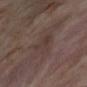The lesion was tiled from a total-body skin photograph and was not biopsied. Located on the right lower leg. A female patient, aged around 55. A roughly 15 mm field-of-view crop from a total-body skin photograph.